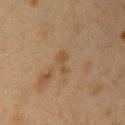• biopsy status: total-body-photography surveillance lesion; no biopsy
• patient: female, aged 38–42
• body site: the arm
• image source: total-body-photography crop, ~15 mm field of view